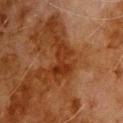Captured during whole-body skin photography for melanoma surveillance; the lesion was not biopsied.
An algorithmic analysis of the crop reported a lesion area of about 7 mm², a shape eccentricity near 0.8, and a symmetry-axis asymmetry near 0.45. The software also gave a border-irregularity rating of about 6.5/10, a color-variation rating of about 4.5/10, and a peripheral color-asymmetry measure near 2.
The lesion is located on the upper back.
The recorded lesion diameter is about 4 mm.
Captured under cross-polarized illumination.
A male patient, roughly 80 years of age.
A 15 mm crop from a total-body photograph taken for skin-cancer surveillance.